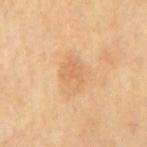Part of a total-body skin-imaging series; this lesion was reviewed on a skin check and was not flagged for biopsy. The lesion-visualizer software estimated a lesion area of about 7 mm² and an eccentricity of roughly 0.7. The analysis additionally found a classifier nevus-likeness of about 0/100 and a lesion-detection confidence of about 100/100. The lesion is on the mid back. The tile uses cross-polarized illumination. A patient aged around 65. A 15 mm close-up tile from a total-body photography series done for melanoma screening. The recorded lesion diameter is about 3.5 mm.